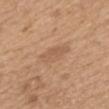notes — catalogued during a skin exam; not biopsied | image-analysis metrics — a mean CIELAB color near L≈57 a*≈19 b*≈33 and about 7 CIELAB-L* units darker than the surrounding skin; a border-irregularity rating of about 3/10 and a color-variation rating of about 1.5/10 | subject — female, aged approximately 70 | size — ~4.5 mm (longest diameter) | body site — the back | image — ~15 mm crop, total-body skin-cancer survey.